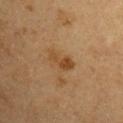workup: imaged on a skin check; not biopsied
patient: male, aged around 60
image: ~15 mm tile from a whole-body skin photo
TBP lesion metrics: a lesion area of about 5.5 mm² and an outline eccentricity of about 0.85 (0 = round, 1 = elongated); a detector confidence of about 100 out of 100 that the crop contains a lesion
lesion diameter: about 3.5 mm
lighting: cross-polarized